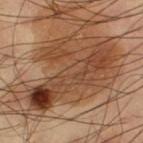Notes:
– notes — imaged on a skin check; not biopsied
– TBP lesion metrics — a footprint of about 40 mm² and a shape-asymmetry score of about 0.55 (0 = symmetric); a mean CIELAB color near L≈43 a*≈21 b*≈32, about 11 CIELAB-L* units darker than the surrounding skin, and a normalized border contrast of about 8.5; border irregularity of about 8.5 on a 0–10 scale and peripheral color asymmetry of about 3; a lesion-detection confidence of about 75/100
– body site — the left thigh
– patient — male, in their 60s
– lighting — cross-polarized
– image — ~15 mm tile from a whole-body skin photo
– lesion diameter — ~11 mm (longest diameter)